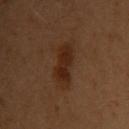Impression: Imaged during a routine full-body skin examination; the lesion was not biopsied and no histopathology is available. Image and clinical context: The subject is a female aged approximately 50. An algorithmic analysis of the crop reported a footprint of about 10 mm², an outline eccentricity of about 0.85 (0 = round, 1 = elongated), and two-axis asymmetry of about 0.25. And it measured an average lesion color of about L≈21 a*≈16 b*≈24 (CIELAB), a lesion–skin lightness drop of about 7, and a lesion-to-skin contrast of about 8 (normalized; higher = more distinct). It also reported border irregularity of about 2.5 on a 0–10 scale and a color-variation rating of about 2.5/10. The software also gave a classifier nevus-likeness of about 80/100 and a lesion-detection confidence of about 100/100. This image is a 15 mm lesion crop taken from a total-body photograph. About 5 mm across. Located on the chest. The tile uses cross-polarized illumination.